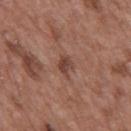Q: Is there a histopathology result?
A: catalogued during a skin exam; not biopsied
Q: Lesion size?
A: ~3 mm (longest diameter)
Q: Who is the patient?
A: male, aged approximately 50
Q: Lesion location?
A: the mid back
Q: What is the imaging modality?
A: ~15 mm crop, total-body skin-cancer survey
Q: How was the tile lit?
A: white-light illumination
Q: What did automated image analysis measure?
A: a lesion area of about 3.5 mm², a shape eccentricity near 0.75, and a shape-asymmetry score of about 0.25 (0 = symmetric); roughly 9 lightness units darker than nearby skin and a normalized lesion–skin contrast near 7.5; border irregularity of about 3 on a 0–10 scale and peripheral color asymmetry of about 1; a classifier nevus-likeness of about 80/100 and a detector confidence of about 100 out of 100 that the crop contains a lesion